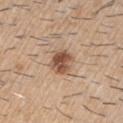biopsy status = total-body-photography surveillance lesion; no biopsy
image = ~15 mm tile from a whole-body skin photo
lighting = white-light
site = the right upper arm
lesion diameter = ~3 mm (longest diameter)
subject = male, aged approximately 60